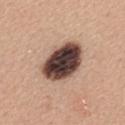The lesion was tiled from a total-body skin photograph and was not biopsied. Approximately 6 mm at its widest. This image is a 15 mm lesion crop taken from a total-body photograph. A female patient in their 40s. The lesion is on the back. This is a white-light tile.